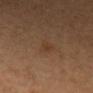Recorded during total-body skin imaging; not selected for excision or biopsy. This is a cross-polarized tile. About 2.5 mm across. A female patient roughly 45 years of age. A 15 mm crop from a total-body photograph taken for skin-cancer surveillance. On the head or neck.